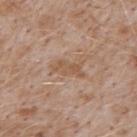Assessment:
Imaged during a routine full-body skin examination; the lesion was not biopsied and no histopathology is available.
Background:
From the upper back. Cropped from a whole-body photographic skin survey; the tile spans about 15 mm. The tile uses white-light illumination. The recorded lesion diameter is about 4 mm. A male patient aged around 50.